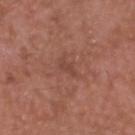The lesion was tiled from a total-body skin photograph and was not biopsied. Captured under white-light illumination. The lesion is located on the head or neck. The patient is a male aged 48 to 52. A roughly 15 mm field-of-view crop from a total-body skin photograph.